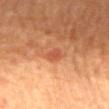notes = catalogued during a skin exam; not biopsied
image source = 15 mm crop, total-body photography
body site = the mid back
subject = male, in their 70s